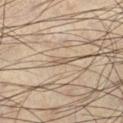workup — total-body-photography surveillance lesion; no biopsy